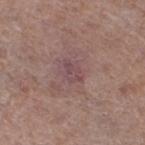A 15 mm close-up extracted from a 3D total-body photography capture.
The subject is a male aged 78–82.
Automated tile analysis of the lesion measured roughly 6 lightness units darker than nearby skin and a normalized border contrast of about 5.5.
The lesion is on the left lower leg.
The lesion's longest dimension is about 2.5 mm.